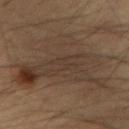Captured during whole-body skin photography for melanoma surveillance; the lesion was not biopsied. This is a cross-polarized tile. A close-up tile cropped from a whole-body skin photograph, about 15 mm across. The lesion is located on the left forearm. A male patient, aged around 60. The recorded lesion diameter is about 10 mm.